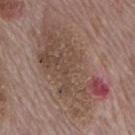{"biopsy_status": "not biopsied; imaged during a skin examination", "site": "mid back", "image": {"source": "total-body photography crop", "field_of_view_mm": 15}, "patient": {"sex": "male", "age_approx": 70}}